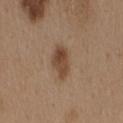Clinical impression:
Captured during whole-body skin photography for melanoma surveillance; the lesion was not biopsied.
Acquisition and patient details:
The total-body-photography lesion software estimated a mean CIELAB color near L≈45 a*≈17 b*≈30, roughly 10 lightness units darker than nearby skin, and a normalized border contrast of about 8. The software also gave a border-irregularity rating of about 2/10, a color-variation rating of about 5/10, and peripheral color asymmetry of about 1.5. And it measured a lesion-detection confidence of about 100/100. About 4 mm across. A 15 mm close-up extracted from a 3D total-body photography capture. Located on the mid back. The tile uses white-light illumination. A female subject, roughly 40 years of age.